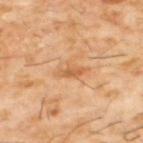Clinical impression:
No biopsy was performed on this lesion — it was imaged during a full skin examination and was not determined to be concerning.
Image and clinical context:
This image is a 15 mm lesion crop taken from a total-body photograph. A male patient aged around 60. Located on the back.